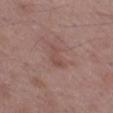Assessment:
Captured during whole-body skin photography for melanoma surveillance; the lesion was not biopsied.
Background:
The lesion is located on the left thigh. The tile uses white-light illumination. The lesion-visualizer software estimated a lesion area of about 4.5 mm². The analysis additionally found a border-irregularity rating of about 4.5/10, a within-lesion color-variation index near 1.5/10, and a peripheral color-asymmetry measure near 0.5. It also reported a lesion-detection confidence of about 100/100. A male subject roughly 50 years of age. A 15 mm close-up tile from a total-body photography series done for melanoma screening. About 3.5 mm across.